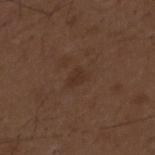Q: What kind of image is this?
A: ~15 mm tile from a whole-body skin photo
Q: What is the anatomic site?
A: the mid back
Q: What lighting was used for the tile?
A: white-light illumination
Q: What is the lesion's diameter?
A: ~2.5 mm (longest diameter)
Q: Who is the patient?
A: male, aged 48–52